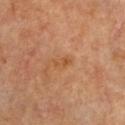Impression:
Recorded during total-body skin imaging; not selected for excision or biopsy.
Clinical summary:
A female patient, aged 63–67. The lesion is on the chest. A close-up tile cropped from a whole-body skin photograph, about 15 mm across. This is a cross-polarized tile. Automated tile analysis of the lesion measured a footprint of about 3 mm² and a shape-asymmetry score of about 0.35 (0 = symmetric). And it measured an average lesion color of about L≈51 a*≈23 b*≈38 (CIELAB), a lesion–skin lightness drop of about 6, and a normalized border contrast of about 5.5. The analysis additionally found border irregularity of about 3 on a 0–10 scale, a color-variation rating of about 1.5/10, and peripheral color asymmetry of about 0.5. The software also gave lesion-presence confidence of about 100/100.